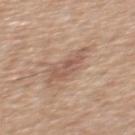workup: catalogued during a skin exam; not biopsied | tile lighting: white-light | anatomic site: the mid back | automated metrics: a lesion color around L≈56 a*≈19 b*≈27 in CIELAB and a lesion-to-skin contrast of about 6 (normalized; higher = more distinct); an automated nevus-likeness rating near 0 out of 100 and a detector confidence of about 100 out of 100 that the crop contains a lesion | image source: ~15 mm tile from a whole-body skin photo | size: ≈4 mm | patient: male, aged approximately 60.